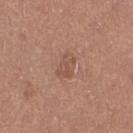The lesion was tiled from a total-body skin photograph and was not biopsied. From the right thigh. Automated tile analysis of the lesion measured a border-irregularity index near 3.5/10, a color-variation rating of about 2/10, and a peripheral color-asymmetry measure near 0.5. Captured under white-light illumination. Cropped from a total-body skin-imaging series; the visible field is about 15 mm. A female subject aged 53 to 57. About 3 mm across.